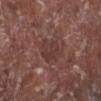biopsy status: no biopsy performed (imaged during a skin exam) | automated lesion analysis: a lesion area of about 6.5 mm² and an outline eccentricity of about 0.45 (0 = round, 1 = elongated); an average lesion color of about L≈36 a*≈21 b*≈21 (CIELAB), a lesion–skin lightness drop of about 6, and a normalized lesion–skin contrast near 5.5; a border-irregularity rating of about 3.5/10, a within-lesion color-variation index near 2.5/10, and a peripheral color-asymmetry measure near 1; a detector confidence of about 85 out of 100 that the crop contains a lesion | patient: male, aged 63 to 67 | imaging modality: ~15 mm tile from a whole-body skin photo | body site: the left lower leg | lesion diameter: ~3 mm (longest diameter) | illumination: white-light.